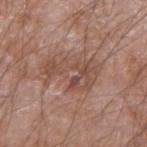Impression:
Recorded during total-body skin imaging; not selected for excision or biopsy.
Clinical summary:
Automated tile analysis of the lesion measured an area of roughly 13 mm², an outline eccentricity of about 0.85 (0 = round, 1 = elongated), and two-axis asymmetry of about 0.6. Cropped from a total-body skin-imaging series; the visible field is about 15 mm. On the right forearm. A male subject aged 58 to 62. Captured under white-light illumination. The lesion's longest dimension is about 5.5 mm.